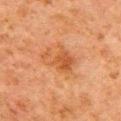follow-up: total-body-photography surveillance lesion; no biopsy
anatomic site: the right upper arm
acquisition: ~15 mm crop, total-body skin-cancer survey
subject: male, aged around 80
lesion diameter: about 4.5 mm